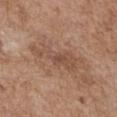Part of a total-body skin-imaging series; this lesion was reviewed on a skin check and was not flagged for biopsy. A female patient aged 73–77. From the chest. Automated tile analysis of the lesion measured an average lesion color of about L≈50 a*≈19 b*≈28 (CIELAB) and a normalized border contrast of about 6. And it measured a classifier nevus-likeness of about 0/100 and lesion-presence confidence of about 100/100. A lesion tile, about 15 mm wide, cut from a 3D total-body photograph. Approximately 6 mm at its widest.